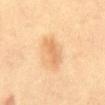patient: female, about 55 years old
lighting: cross-polarized
location: the front of the torso
image source: ~15 mm tile from a whole-body skin photo
lesion size: about 4 mm
image-analysis metrics: a mean CIELAB color near L≈61 a*≈19 b*≈37, about 8 CIELAB-L* units darker than the surrounding skin, and a normalized border contrast of about 6; radial color variation of about 0.5